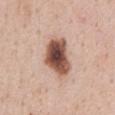Assessment:
The lesion was photographed on a routine skin check and not biopsied; there is no pathology result.
Context:
The patient is a female roughly 45 years of age. A 15 mm close-up tile from a total-body photography series done for melanoma screening. The lesion is located on the abdomen.